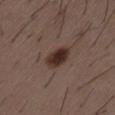Measured at roughly 3.5 mm in maximum diameter.
On the left thigh.
This is a white-light tile.
A male patient approximately 50 years of age.
Automated image analysis of the tile measured roughly 13 lightness units darker than nearby skin and a lesion-to-skin contrast of about 12 (normalized; higher = more distinct). The analysis additionally found a nevus-likeness score of about 100/100 and a detector confidence of about 100 out of 100 that the crop contains a lesion.
This image is a 15 mm lesion crop taken from a total-body photograph.